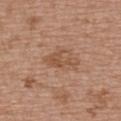{"biopsy_status": "not biopsied; imaged during a skin examination", "patient": {"sex": "female", "age_approx": 60}, "image": {"source": "total-body photography crop", "field_of_view_mm": 15}, "site": "upper back", "lesion_size": {"long_diameter_mm_approx": 4.0}}